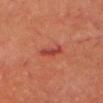Findings:
– follow-up · no biopsy performed (imaged during a skin exam)
– subject · male, roughly 70 years of age
– TBP lesion metrics · a lesion area of about 4 mm², an outline eccentricity of about 0.9 (0 = round, 1 = elongated), and two-axis asymmetry of about 0.3; a mean CIELAB color near L≈37 a*≈30 b*≈25, roughly 8 lightness units darker than nearby skin, and a lesion-to-skin contrast of about 7 (normalized; higher = more distinct); a border-irregularity index near 4/10 and radial color variation of about 0.5
– illumination · cross-polarized illumination
– acquisition · ~15 mm tile from a whole-body skin photo
– location · the head or neck